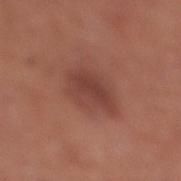biopsy status=catalogued during a skin exam; not biopsied | tile lighting=white-light | location=the right lower leg | subject=male, about 70 years old | acquisition=~15 mm tile from a whole-body skin photo.